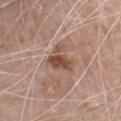Part of a total-body skin-imaging series; this lesion was reviewed on a skin check and was not flagged for biopsy.
The lesion is on the chest.
The lesion-visualizer software estimated an average lesion color of about L≈49 a*≈21 b*≈27 (CIELAB), roughly 11 lightness units darker than nearby skin, and a normalized border contrast of about 8.5.
Approximately 3.5 mm at its widest.
The tile uses white-light illumination.
A 15 mm crop from a total-body photograph taken for skin-cancer surveillance.
The patient is a male aged 78–82.